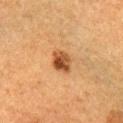Acquisition and patient details: From the arm. About 3.5 mm across. A male subject, aged around 50. Captured under cross-polarized illumination. A lesion tile, about 15 mm wide, cut from a 3D total-body photograph.